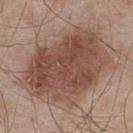body site — the upper back | patient — male, in their mid- to late 50s | image-analysis metrics — a footprint of about 60 mm², an outline eccentricity of about 0.7 (0 = round, 1 = elongated), and two-axis asymmetry of about 0.2; border irregularity of about 2.5 on a 0–10 scale and peripheral color asymmetry of about 2; a lesion-detection confidence of about 100/100 | image source — 15 mm crop, total-body photography | tile lighting — white-light | lesion size — ≈10 mm.